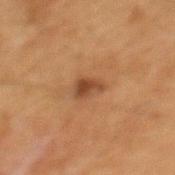Image and clinical context: This is a cross-polarized tile. The subject is a male approximately 65 years of age. A region of skin cropped from a whole-body photographic capture, roughly 15 mm wide. Approximately 2.5 mm at its widest. From the mid back.